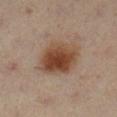workup: catalogued during a skin exam; not biopsied | illumination: cross-polarized | subject: female, in their 40s | site: the leg | image: 15 mm crop, total-body photography | size: ≈5 mm.